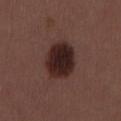| key | value |
|---|---|
| follow-up | catalogued during a skin exam; not biopsied |
| size | ~5 mm (longest diameter) |
| imaging modality | ~15 mm crop, total-body skin-cancer survey |
| subject | male, in their 30s |
| lighting | white-light illumination |
| TBP lesion metrics | a footprint of about 16 mm², an eccentricity of roughly 0.6, and a shape-asymmetry score of about 0.15 (0 = symmetric); a lesion color around L≈24 a*≈18 b*≈18 in CIELAB, roughly 13 lightness units darker than nearby skin, and a normalized lesion–skin contrast near 13.5; a nevus-likeness score of about 90/100 |
| anatomic site | the back |